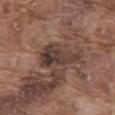biopsy status — total-body-photography surveillance lesion; no biopsy
anatomic site — the abdomen
lesion diameter — ≈5 mm
patient — male, approximately 75 years of age
acquisition — total-body-photography crop, ~15 mm field of view
illumination — white-light illumination